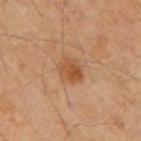Recorded during total-body skin imaging; not selected for excision or biopsy.
A male patient, aged 63 to 67.
Cropped from a total-body skin-imaging series; the visible field is about 15 mm.
On the mid back.
Imaged with cross-polarized lighting.